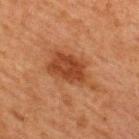{
  "biopsy_status": "not biopsied; imaged during a skin examination",
  "image": {
    "source": "total-body photography crop",
    "field_of_view_mm": 15
  },
  "patient": {
    "sex": "female",
    "age_approx": 40
  },
  "lesion_size": {
    "long_diameter_mm_approx": 6.5
  },
  "lighting": "cross-polarized",
  "site": "back"
}